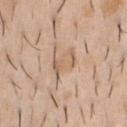Clinical impression:
The lesion was photographed on a routine skin check and not biopsied; there is no pathology result.
Clinical summary:
A male patient aged approximately 40. The tile uses white-light illumination. The lesion is located on the chest. A lesion tile, about 15 mm wide, cut from a 3D total-body photograph. Measured at roughly 4 mm in maximum diameter. The lesion-visualizer software estimated internal color variation of about 3.5 on a 0–10 scale and peripheral color asymmetry of about 1.